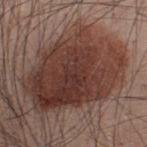Captured during whole-body skin photography for melanoma surveillance; the lesion was not biopsied.
This is a white-light tile.
The total-body-photography lesion software estimated a lesion area of about 60 mm² and a symmetry-axis asymmetry near 0.2. It also reported a mean CIELAB color near L≈37 a*≈20 b*≈23, roughly 13 lightness units darker than nearby skin, and a lesion-to-skin contrast of about 10.5 (normalized; higher = more distinct). It also reported a nevus-likeness score of about 95/100.
Approximately 11 mm at its widest.
A male subject, in their mid-30s.
On the upper back.
A close-up tile cropped from a whole-body skin photograph, about 15 mm across.